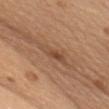The lesion was photographed on a routine skin check and not biopsied; there is no pathology result. The lesion's longest dimension is about 5.5 mm. A female patient aged 58–62. The lesion is located on the chest. A 15 mm close-up extracted from a 3D total-body photography capture. This is a white-light tile. The lesion-visualizer software estimated a lesion color around L≈49 a*≈20 b*≈31 in CIELAB, roughly 8 lightness units darker than nearby skin, and a lesion-to-skin contrast of about 6 (normalized; higher = more distinct). The analysis additionally found border irregularity of about 5.5 on a 0–10 scale, a color-variation rating of about 4/10, and a peripheral color-asymmetry measure near 1.5.